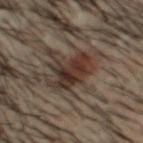Q: Is there a histopathology result?
A: catalogued during a skin exam; not biopsied
Q: What kind of image is this?
A: total-body-photography crop, ~15 mm field of view
Q: What are the patient's age and sex?
A: male, in their 30s
Q: Automated lesion metrics?
A: a shape eccentricity near 0.5; a border-irregularity index near 4/10, internal color variation of about 5.5 on a 0–10 scale, and peripheral color asymmetry of about 2
Q: How was the tile lit?
A: cross-polarized
Q: Lesion location?
A: the head or neck
Q: What is the lesion's diameter?
A: ≈5 mm